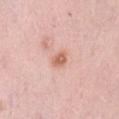| key | value |
|---|---|
| notes | total-body-photography surveillance lesion; no biopsy |
| image | ~15 mm crop, total-body skin-cancer survey |
| site | the abdomen |
| subject | female, aged approximately 40 |
| tile lighting | white-light illumination |